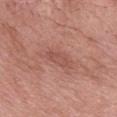The lesion was tiled from a total-body skin photograph and was not biopsied.
The lesion is located on the chest.
A close-up tile cropped from a whole-body skin photograph, about 15 mm across.
A female subject roughly 70 years of age.
Automated image analysis of the tile measured a lesion color around L≈51 a*≈25 b*≈27 in CIELAB and about 6 CIELAB-L* units darker than the surrounding skin. The analysis additionally found a within-lesion color-variation index near 1/10 and a peripheral color-asymmetry measure near 0. And it measured a classifier nevus-likeness of about 0/100 and lesion-presence confidence of about 100/100.